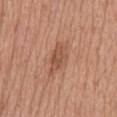A male patient aged 73–77. On the abdomen. A 15 mm close-up extracted from a 3D total-body photography capture. Automated tile analysis of the lesion measured an eccentricity of roughly 0.85 and a symmetry-axis asymmetry near 0.3. And it measured a mean CIELAB color near L≈52 a*≈23 b*≈32 and a lesion-to-skin contrast of about 6.5 (normalized; higher = more distinct). It also reported a within-lesion color-variation index near 2/10 and peripheral color asymmetry of about 0.5.